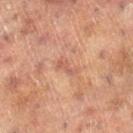Notes:
* follow-up — total-body-photography surveillance lesion; no biopsy